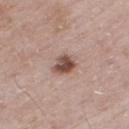The lesion was photographed on a routine skin check and not biopsied; there is no pathology result. The lesion is located on the right thigh. A male patient, in their 60s. A close-up tile cropped from a whole-body skin photograph, about 15 mm across. Longest diameter approximately 2.5 mm. Imaged with white-light lighting.